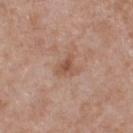Assessment:
This lesion was catalogued during total-body skin photography and was not selected for biopsy.
Clinical summary:
The patient is a male roughly 50 years of age. The lesion is on the chest. A region of skin cropped from a whole-body photographic capture, roughly 15 mm wide.